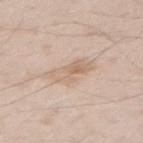Automated tile analysis of the lesion measured a border-irregularity rating of about 4.5/10 and a color-variation rating of about 5/10.
A close-up tile cropped from a whole-body skin photograph, about 15 mm across.
From the right thigh.
The patient is a male aged 63 to 67.
About 4.5 mm across.